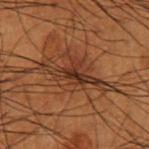Imaged during a routine full-body skin examination; the lesion was not biopsied and no histopathology is available.
About 7.5 mm across.
A 15 mm close-up extracted from a 3D total-body photography capture.
A male patient, aged 48–52.
Captured under cross-polarized illumination.
The lesion is located on the left forearm.